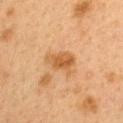Impression:
The lesion was photographed on a routine skin check and not biopsied; there is no pathology result.
Image and clinical context:
Imaged with cross-polarized lighting. Located on the back. Cropped from a total-body skin-imaging series; the visible field is about 15 mm. Measured at roughly 4 mm in maximum diameter. A female patient, approximately 40 years of age. The total-body-photography lesion software estimated a footprint of about 7 mm², an eccentricity of roughly 0.75, and a symmetry-axis asymmetry near 0.35. And it measured a mean CIELAB color near L≈48 a*≈20 b*≈36, a lesion–skin lightness drop of about 9, and a normalized lesion–skin contrast near 7. It also reported lesion-presence confidence of about 100/100.